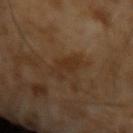Notes:
– biopsy status · catalogued during a skin exam; not biopsied
– size · ≈4 mm
– lighting · cross-polarized
– image · ~15 mm crop, total-body skin-cancer survey
– patient · male, in their mid- to late 60s